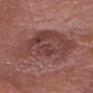workup: catalogued during a skin exam; not biopsied | imaging modality: ~15 mm crop, total-body skin-cancer survey | illumination: white-light illumination | diameter: ≈10 mm | image-analysis metrics: an automated nevus-likeness rating near 5 out of 100 and a lesion-detection confidence of about 100/100 | body site: the head or neck | patient: male, aged approximately 80.